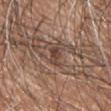Q: Was a biopsy performed?
A: total-body-photography surveillance lesion; no biopsy
Q: How was this image acquired?
A: ~15 mm crop, total-body skin-cancer survey
Q: What are the patient's age and sex?
A: male, approximately 45 years of age
Q: Lesion size?
A: about 3 mm
Q: What did automated image analysis measure?
A: an area of roughly 3 mm², a shape eccentricity near 0.85, and a symmetry-axis asymmetry near 0.35; an average lesion color of about L≈42 a*≈18 b*≈25 (CIELAB); border irregularity of about 3.5 on a 0–10 scale, a within-lesion color-variation index near 3/10, and radial color variation of about 0.5; a lesion-detection confidence of about 60/100
Q: Illumination type?
A: white-light illumination
Q: What is the anatomic site?
A: the chest